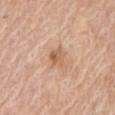Impression: The lesion was tiled from a total-body skin photograph and was not biopsied. Clinical summary: The lesion is located on the chest. The recorded lesion diameter is about 2.5 mm. Captured under white-light illumination. A male patient about 65 years old. The lesion-visualizer software estimated a shape eccentricity near 0.6 and two-axis asymmetry of about 0.25. And it measured a mean CIELAB color near L≈60 a*≈21 b*≈33. The analysis additionally found a detector confidence of about 100 out of 100 that the crop contains a lesion. A lesion tile, about 15 mm wide, cut from a 3D total-body photograph.